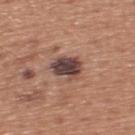No biopsy was performed on this lesion — it was imaged during a full skin examination and was not determined to be concerning. A region of skin cropped from a whole-body photographic capture, roughly 15 mm wide. The recorded lesion diameter is about 4 mm. The lesion is located on the back. This is a white-light tile. The patient is a male approximately 45 years of age. The lesion-visualizer software estimated a lesion color around L≈43 a*≈17 b*≈22 in CIELAB, about 15 CIELAB-L* units darker than the surrounding skin, and a normalized lesion–skin contrast near 12.5. The software also gave a border-irregularity rating of about 2.5/10, a color-variation rating of about 5.5/10, and peripheral color asymmetry of about 1.5.